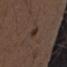Part of a total-body skin-imaging series; this lesion was reviewed on a skin check and was not flagged for biopsy. Captured under white-light illumination. A lesion tile, about 15 mm wide, cut from a 3D total-body photograph. The lesion is on the arm. The subject is a male about 50 years old. The total-body-photography lesion software estimated a lesion area of about 2 mm² and an eccentricity of roughly 0.9. The analysis additionally found an average lesion color of about L≈30 a*≈13 b*≈20 (CIELAB) and about 8 CIELAB-L* units darker than the surrounding skin. The software also gave border irregularity of about 3.5 on a 0–10 scale, a within-lesion color-variation index near 0/10, and radial color variation of about 0. And it measured an automated nevus-likeness rating near 55 out of 100 and lesion-presence confidence of about 100/100.